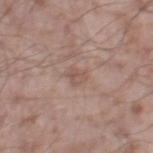This lesion was catalogued during total-body skin photography and was not selected for biopsy. A 15 mm close-up tile from a total-body photography series done for melanoma screening. From the leg. A male subject, aged around 55.